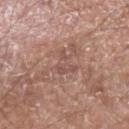Imaged during a routine full-body skin examination; the lesion was not biopsied and no histopathology is available.
An algorithmic analysis of the crop reported an area of roughly 5.5 mm² and two-axis asymmetry of about 0.55.
A lesion tile, about 15 mm wide, cut from a 3D total-body photograph.
The lesion is on the left forearm.
Imaged with white-light lighting.
Approximately 3.5 mm at its widest.
A male patient aged 53 to 57.